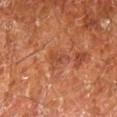<case>
  <biopsy_status>not biopsied; imaged during a skin examination</biopsy_status>
  <lesion_size>
    <long_diameter_mm_approx>3.0</long_diameter_mm_approx>
  </lesion_size>
  <automated_metrics>
    <cielab_L>45</cielab_L>
    <cielab_a>27</cielab_a>
    <cielab_b>33</cielab_b>
    <vs_skin_darker_L>6.0</vs_skin_darker_L>
    <vs_skin_contrast_norm>5.0</vs_skin_contrast_norm>
    <nevus_likeness_0_100>0</nevus_likeness_0_100>
    <lesion_detection_confidence_0_100>100</lesion_detection_confidence_0_100>
  </automated_metrics>
  <patient>
    <sex>male</sex>
    <age_approx>65</age_approx>
  </patient>
  <site>left lower leg</site>
  <image>
    <source>total-body photography crop</source>
    <field_of_view_mm>15</field_of_view_mm>
  </image>
  <lighting>cross-polarized</lighting>
</case>